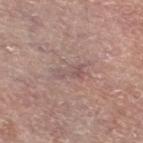notes = total-body-photography surveillance lesion; no biopsy | subject = male, aged approximately 55 | size = ≈4 mm | location = the left lower leg | imaging modality = total-body-photography crop, ~15 mm field of view.